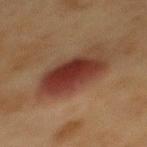Q: Was a biopsy performed?
A: no biopsy performed (imaged during a skin exam)
Q: What are the patient's age and sex?
A: female, aged around 40
Q: What is the lesion's diameter?
A: ≈7 mm
Q: How was this image acquired?
A: ~15 mm crop, total-body skin-cancer survey
Q: Lesion location?
A: the mid back
Q: What did automated image analysis measure?
A: an average lesion color of about L≈31 a*≈21 b*≈24 (CIELAB) and roughly 11 lightness units darker than nearby skin; a border-irregularity index near 2/10, a within-lesion color-variation index near 6.5/10, and radial color variation of about 2
Q: How was the tile lit?
A: cross-polarized